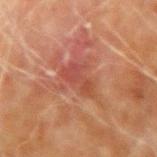Captured during whole-body skin photography for melanoma surveillance; the lesion was not biopsied. Longest diameter approximately 4 mm. Cropped from a whole-body photographic skin survey; the tile spans about 15 mm. A male patient, aged approximately 70. On the left upper arm.